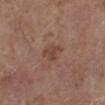Background: Imaged with white-light lighting. On the leg. Automated image analysis of the tile measured an area of roughly 5 mm² and a symmetry-axis asymmetry near 0.3. The analysis additionally found a border-irregularity index near 3/10, a color-variation rating of about 2.5/10, and radial color variation of about 1. And it measured a nevus-likeness score of about 0/100 and lesion-presence confidence of about 100/100. A lesion tile, about 15 mm wide, cut from a 3D total-body photograph. Longest diameter approximately 3 mm. A female subject, in their mid-60s.